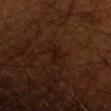The lesion was tiled from a total-body skin photograph and was not biopsied. A male subject, in their 70s. Longest diameter approximately 3 mm. The tile uses cross-polarized illumination. A 15 mm crop from a total-body photograph taken for skin-cancer surveillance. On the right upper arm.